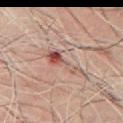Clinical impression: The lesion was tiled from a total-body skin photograph and was not biopsied. Background: A region of skin cropped from a whole-body photographic capture, roughly 15 mm wide. Located on the chest. A male subject, approximately 60 years of age. The lesion's longest dimension is about 5 mm.